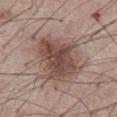notes = catalogued during a skin exam; not biopsied | anatomic site = the abdomen | lesion diameter = about 7 mm | tile lighting = white-light illumination | patient = male, aged 53–57 | image = ~15 mm tile from a whole-body skin photo.